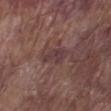Recorded during total-body skin imaging; not selected for excision or biopsy. A male subject, aged 78–82. Approximately 3.5 mm at its widest. This is a white-light tile. An algorithmic analysis of the crop reported a mean CIELAB color near L≈37 a*≈19 b*≈16, roughly 7 lightness units darker than nearby skin, and a normalized lesion–skin contrast near 7.5. It also reported a border-irregularity index near 3.5/10 and internal color variation of about 1.5 on a 0–10 scale. And it measured a classifier nevus-likeness of about 0/100 and a detector confidence of about 80 out of 100 that the crop contains a lesion. The lesion is on the left lower leg. A close-up tile cropped from a whole-body skin photograph, about 15 mm across.